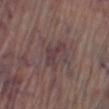notes: imaged on a skin check; not biopsied | anatomic site: the right lower leg | patient: male, aged approximately 70 | imaging modality: ~15 mm crop, total-body skin-cancer survey | lighting: white-light | lesion size: ~4 mm (longest diameter).